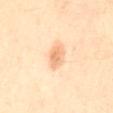This lesion was catalogued during total-body skin photography and was not selected for biopsy.